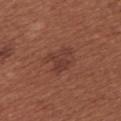Q: Was this lesion biopsied?
A: catalogued during a skin exam; not biopsied
Q: Lesion location?
A: the upper back
Q: What did automated image analysis measure?
A: a detector confidence of about 100 out of 100 that the crop contains a lesion
Q: Who is the patient?
A: female, aged 63–67
Q: Illumination type?
A: white-light
Q: What is the lesion's diameter?
A: ~3.5 mm (longest diameter)
Q: What is the imaging modality?
A: total-body-photography crop, ~15 mm field of view